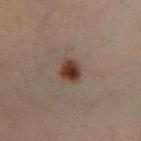notes = imaged on a skin check; not biopsied | patient = male, aged around 55 | image source = total-body-photography crop, ~15 mm field of view | body site = the right lower leg.